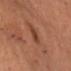The patient is a male aged approximately 60. A roughly 15 mm field-of-view crop from a total-body skin photograph. On the front of the torso.